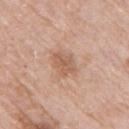Imaged during a routine full-body skin examination; the lesion was not biopsied and no histopathology is available.
A 15 mm close-up extracted from a 3D total-body photography capture.
A female patient approximately 70 years of age.
The lesion is located on the right upper arm.